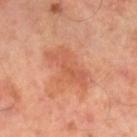Assessment: The lesion was tiled from a total-body skin photograph and was not biopsied. Clinical summary: A male subject, in their 50s. A 15 mm crop from a total-body photograph taken for skin-cancer surveillance. The total-body-photography lesion software estimated a footprint of about 15 mm², an outline eccentricity of about 0.85 (0 = round, 1 = elongated), and a symmetry-axis asymmetry near 0.35. The tile uses cross-polarized illumination. Measured at roughly 6 mm in maximum diameter. From the left leg.